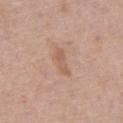{"biopsy_status": "not biopsied; imaged during a skin examination", "lighting": "white-light", "patient": {"sex": "male", "age_approx": 80}, "site": "abdomen", "image": {"source": "total-body photography crop", "field_of_view_mm": 15}}